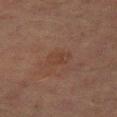Q: Is there a histopathology result?
A: imaged on a skin check; not biopsied
Q: Where on the body is the lesion?
A: the left thigh
Q: How was this image acquired?
A: ~15 mm tile from a whole-body skin photo
Q: Who is the patient?
A: male, aged 68 to 72
Q: How large is the lesion?
A: ≈3 mm